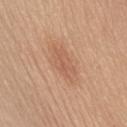  biopsy_status: not biopsied; imaged during a skin examination
  patient:
    sex: female
    age_approx: 45
  site: chest
  lighting: white-light
  lesion_size:
    long_diameter_mm_approx: 5.5
  image:
    source: total-body photography crop
    field_of_view_mm: 15